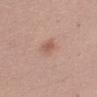Impression: Recorded during total-body skin imaging; not selected for excision or biopsy. Image and clinical context: The lesion is on the left upper arm. Cropped from a whole-body photographic skin survey; the tile spans about 15 mm. A female subject, aged 48–52. Automated image analysis of the tile measured a within-lesion color-variation index near 3.5/10. Longest diameter approximately 4.5 mm. Imaged with white-light lighting.